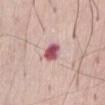The lesion was tiled from a total-body skin photograph and was not biopsied. This is a white-light tile. The patient is a male roughly 75 years of age. The lesion is on the front of the torso. The recorded lesion diameter is about 3 mm. A roughly 15 mm field-of-view crop from a total-body skin photograph.